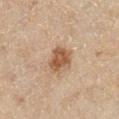subject = female, aged 58–62
image source = total-body-photography crop, ~15 mm field of view
tile lighting = cross-polarized illumination
TBP lesion metrics = an area of roughly 7 mm² and a shape eccentricity near 0.55; a mean CIELAB color near L≈49 a*≈18 b*≈31, roughly 12 lightness units darker than nearby skin, and a normalized border contrast of about 9; a border-irregularity rating of about 2.5/10, a within-lesion color-variation index near 2.5/10, and a peripheral color-asymmetry measure near 1; an automated nevus-likeness rating near 80 out of 100 and lesion-presence confidence of about 100/100
body site = the right lower leg